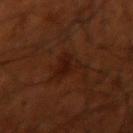The lesion was tiled from a total-body skin photograph and was not biopsied.